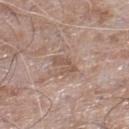No biopsy was performed on this lesion — it was imaged during a full skin examination and was not determined to be concerning.
This is a white-light tile.
A male subject aged 73 to 77.
The lesion-visualizer software estimated a border-irregularity rating of about 4.5/10, a color-variation rating of about 1.5/10, and peripheral color asymmetry of about 0.5.
A close-up tile cropped from a whole-body skin photograph, about 15 mm across.
From the right lower leg.